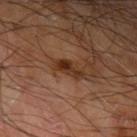  biopsy_status: not biopsied; imaged during a skin examination
  lighting: cross-polarized
  lesion_size:
    long_diameter_mm_approx: 3.5
  site: arm
  patient:
    sex: male
    age_approx: 65
  image:
    source: total-body photography crop
    field_of_view_mm: 15
  automated_metrics:
    cielab_L: 32
    cielab_a: 21
    cielab_b: 30
    vs_skin_darker_L: 11.0